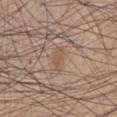Notes:
- workup: total-body-photography surveillance lesion; no biopsy
- anatomic site: the front of the torso
- subject: male, aged 33 to 37
- tile lighting: white-light illumination
- acquisition: total-body-photography crop, ~15 mm field of view
- image-analysis metrics: an area of roughly 3.5 mm², an outline eccentricity of about 0.85 (0 = round, 1 = elongated), and a shape-asymmetry score of about 0.35 (0 = symmetric); an average lesion color of about L≈54 a*≈16 b*≈29 (CIELAB) and roughly 5 lightness units darker than nearby skin; a color-variation rating of about 1/10 and a peripheral color-asymmetry measure near 0; a classifier nevus-likeness of about 25/100 and lesion-presence confidence of about 90/100